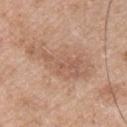The lesion was photographed on a routine skin check and not biopsied; there is no pathology result. Cropped from a whole-body photographic skin survey; the tile spans about 15 mm. A male patient, in their 50s. The lesion is located on the chest.